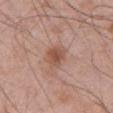{
  "biopsy_status": "not biopsied; imaged during a skin examination",
  "automated_metrics": {
    "cielab_L": 52,
    "cielab_a": 22,
    "cielab_b": 28,
    "vs_skin_darker_L": 10.0,
    "vs_skin_contrast_norm": 7.0,
    "color_variation_0_10": 4.0,
    "peripheral_color_asymmetry": 1.0
  },
  "patient": {
    "sex": "male",
    "age_approx": 70
  },
  "image": {
    "source": "total-body photography crop",
    "field_of_view_mm": 15
  },
  "lighting": "white-light",
  "lesion_size": {
    "long_diameter_mm_approx": 3.0
  },
  "site": "abdomen"
}